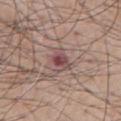Q: Was a biopsy performed?
A: catalogued during a skin exam; not biopsied
Q: Where on the body is the lesion?
A: the chest
Q: What are the patient's age and sex?
A: male, roughly 55 years of age
Q: How was this image acquired?
A: total-body-photography crop, ~15 mm field of view